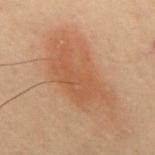Imaged during a routine full-body skin examination; the lesion was not biopsied and no histopathology is available.
A 15 mm close-up extracted from a 3D total-body photography capture.
Imaged with cross-polarized lighting.
A male subject aged approximately 55.
Located on the back.